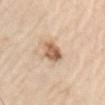{"biopsy_status": "not biopsied; imaged during a skin examination", "lighting": "white-light", "site": "left upper arm", "image": {"source": "total-body photography crop", "field_of_view_mm": 15}, "patient": {"sex": "male", "age_approx": 80}, "automated_metrics": {"cielab_L": 62, "cielab_a": 18, "cielab_b": 34, "vs_skin_darker_L": 14.0, "vs_skin_contrast_norm": 9.0}, "lesion_size": {"long_diameter_mm_approx": 3.5}}